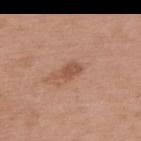– workup · no biopsy performed (imaged during a skin exam)
– lesion size · about 2.5 mm
– subject · female, roughly 70 years of age
– site · the upper back
– imaging modality · ~15 mm crop, total-body skin-cancer survey
– illumination · white-light